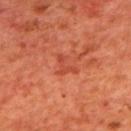location=the upper back; image source=15 mm crop, total-body photography; illumination=cross-polarized illumination; size=≈3 mm; subject=male, aged approximately 65.